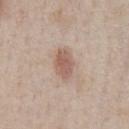workup: no biopsy performed (imaged during a skin exam); acquisition: 15 mm crop, total-body photography; body site: the chest; patient: male, about 60 years old.